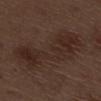Q: Was this lesion biopsied?
A: imaged on a skin check; not biopsied
Q: What lighting was used for the tile?
A: white-light
Q: Who is the patient?
A: male, aged 68 to 72
Q: What did automated image analysis measure?
A: a footprint of about 28 mm², a shape eccentricity near 0.9, and a symmetry-axis asymmetry near 0.4; an average lesion color of about L≈26 a*≈15 b*≈21 (CIELAB), about 6 CIELAB-L* units darker than the surrounding skin, and a normalized border contrast of about 6.5; a border-irregularity rating of about 7/10, a color-variation rating of about 3.5/10, and a peripheral color-asymmetry measure near 1; a classifier nevus-likeness of about 0/100 and a lesion-detection confidence of about 100/100
Q: What is the anatomic site?
A: the right thigh
Q: What is the imaging modality?
A: ~15 mm tile from a whole-body skin photo
Q: What is the lesion's diameter?
A: about 9 mm